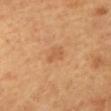Findings:
- biopsy status: total-body-photography surveillance lesion; no biopsy
- image: total-body-photography crop, ~15 mm field of view
- subject: female, in their 60s
- location: the mid back
- TBP lesion metrics: a footprint of about 3 mm² and an outline eccentricity of about 0.8 (0 = round, 1 = elongated); an average lesion color of about L≈59 a*≈24 b*≈41 (CIELAB), a lesion–skin lightness drop of about 7, and a lesion-to-skin contrast of about 5 (normalized; higher = more distinct); internal color variation of about 0.5 on a 0–10 scale; a nevus-likeness score of about 0/100 and lesion-presence confidence of about 100/100
- illumination: cross-polarized
- lesion size: ~2.5 mm (longest diameter)